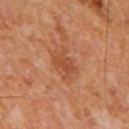notes = total-body-photography surveillance lesion; no biopsy | patient = male, aged 63 to 67 | diameter = ≈3.5 mm | automated lesion analysis = a lesion color around L≈47 a*≈26 b*≈35 in CIELAB and a normalized lesion–skin contrast near 6; an automated nevus-likeness rating near 0 out of 100 | body site = the mid back | imaging modality = 15 mm crop, total-body photography | tile lighting = cross-polarized illumination.